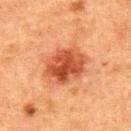Assessment:
Part of a total-body skin-imaging series; this lesion was reviewed on a skin check and was not flagged for biopsy.
Clinical summary:
The lesion's longest dimension is about 5.5 mm. A region of skin cropped from a whole-body photographic capture, roughly 15 mm wide. This is a cross-polarized tile. Automated image analysis of the tile measured an area of roughly 15 mm², an outline eccentricity of about 0.65 (0 = round, 1 = elongated), and a symmetry-axis asymmetry near 0.15. And it measured a mean CIELAB color near L≈41 a*≈28 b*≈33 and a lesion-to-skin contrast of about 9.5 (normalized; higher = more distinct). It also reported internal color variation of about 5 on a 0–10 scale and peripheral color asymmetry of about 1.5. And it measured an automated nevus-likeness rating near 95 out of 100. From the back. A male patient, aged around 50.